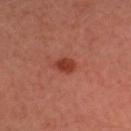Q: Was a biopsy performed?
A: no biopsy performed (imaged during a skin exam)
Q: What is the lesion's diameter?
A: ≈2.5 mm
Q: What are the patient's age and sex?
A: male, in their mid- to late 30s
Q: How was the tile lit?
A: cross-polarized
Q: What is the anatomic site?
A: the head or neck
Q: What is the imaging modality?
A: ~15 mm crop, total-body skin-cancer survey